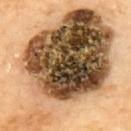image source: ~15 mm tile from a whole-body skin photo
site: the back
lesion size: ~17 mm (longest diameter)